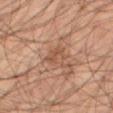Case summary:
- workup · catalogued during a skin exam; not biopsied
- anatomic site · the right thigh
- acquisition · ~15 mm crop, total-body skin-cancer survey
- patient · male, in their mid-50s